The lesion is on the mid back; a roughly 15 mm field-of-view crop from a total-body skin photograph; about 6 mm across; the tile uses white-light illumination; the patient is a male aged around 65; An algorithmic analysis of the crop reported a mean CIELAB color near L≈46 a*≈18 b*≈18 and a lesion–skin lightness drop of about 15.
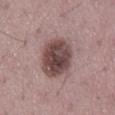  diagnosis:
    histopathology: dysplastic (Clark) nevus
    malignancy: benign
    taxonomic_path:
      - Benign
      - Benign melanocytic proliferations
      - Nevus
      - Nevus, Atypical, Dysplastic, or Clark